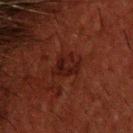Clinical summary:
A male patient, aged around 50. Located on the back. The total-body-photography lesion software estimated a footprint of about 6.5 mm², an outline eccentricity of about 0.65 (0 = round, 1 = elongated), and two-axis asymmetry of about 0.4. The software also gave an average lesion color of about L≈15 a*≈20 b*≈18 (CIELAB), roughly 5 lightness units darker than nearby skin, and a normalized border contrast of about 7. A region of skin cropped from a whole-body photographic capture, roughly 15 mm wide. The tile uses cross-polarized illumination. Measured at roughly 3.5 mm in maximum diameter.